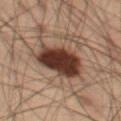The lesion is on the left thigh. A roughly 15 mm field-of-view crop from a total-body skin photograph. The patient is a male aged 53 to 57. About 6 mm across. Imaged with cross-polarized lighting.